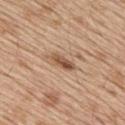Assessment:
The lesion was tiled from a total-body skin photograph and was not biopsied.
Image and clinical context:
The lesion is on the back. A male patient roughly 70 years of age. This image is a 15 mm lesion crop taken from a total-body photograph.